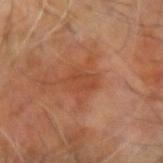workup: no biopsy performed (imaged during a skin exam); patient: male, aged approximately 60; site: the right arm; acquisition: 15 mm crop, total-body photography.